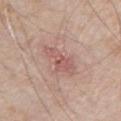Captured during whole-body skin photography for melanoma surveillance; the lesion was not biopsied. A 15 mm close-up tile from a total-body photography series done for melanoma screening. On the chest. This is a white-light tile. A male patient in their 80s.